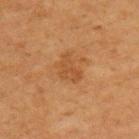{
  "patient": {
    "sex": "male",
    "age_approx": 65
  },
  "lesion_size": {
    "long_diameter_mm_approx": 3.5
  },
  "automated_metrics": {
    "cielab_L": 43,
    "cielab_a": 21,
    "cielab_b": 36,
    "vs_skin_darker_L": 6.0,
    "vs_skin_contrast_norm": 5.5,
    "border_irregularity_0_10": 4.5,
    "color_variation_0_10": 2.0,
    "peripheral_color_asymmetry": 0.5,
    "nevus_likeness_0_100": 5,
    "lesion_detection_confidence_0_100": 100
  },
  "lighting": "cross-polarized",
  "site": "back",
  "image": {
    "source": "total-body photography crop",
    "field_of_view_mm": 15
  }
}